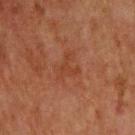<case>
<biopsy_status>not biopsied; imaged during a skin examination</biopsy_status>
<patient>
  <sex>male</sex>
  <age_approx>65</age_approx>
</patient>
<image>
  <source>total-body photography crop</source>
  <field_of_view_mm>15</field_of_view_mm>
</image>
<site>chest</site>
<automated_metrics>
  <cielab_L>33</cielab_L>
  <cielab_a>21</cielab_a>
  <cielab_b>28</cielab_b>
  <vs_skin_darker_L>4.0</vs_skin_darker_L>
  <border_irregularity_0_10>6.0</border_irregularity_0_10>
  <peripheral_color_asymmetry>0.5</peripheral_color_asymmetry>
  <nevus_likeness_0_100>0</nevus_likeness_0_100>
  <lesion_detection_confidence_0_100>100</lesion_detection_confidence_0_100>
</automated_metrics>
</case>